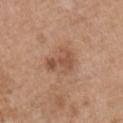About 4 mm across.
This is a white-light tile.
The total-body-photography lesion software estimated a lesion–skin lightness drop of about 9 and a lesion-to-skin contrast of about 6.5 (normalized; higher = more distinct). It also reported a nevus-likeness score of about 5/100 and lesion-presence confidence of about 100/100.
The subject is a male aged approximately 65.
From the chest.
A lesion tile, about 15 mm wide, cut from a 3D total-body photograph.